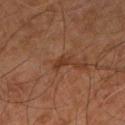workup: imaged on a skin check; not biopsied | lighting: cross-polarized illumination | automated metrics: a footprint of about 3 mm², an eccentricity of roughly 0.85, and a shape-asymmetry score of about 0.3 (0 = symmetric); border irregularity of about 3 on a 0–10 scale, a color-variation rating of about 0.5/10, and radial color variation of about 0 | anatomic site: the right leg | image source: ~15 mm crop, total-body skin-cancer survey | patient: male, aged 58–62 | size: about 2.5 mm.